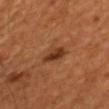biopsy_status: not biopsied; imaged during a skin examination
site: front of the torso
patient:
  sex: male
  age_approx: 45
image:
  source: total-body photography crop
  field_of_view_mm: 15